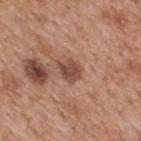<record>
  <biopsy_status>not biopsied; imaged during a skin examination</biopsy_status>
  <patient>
    <sex>male</sex>
    <age_approx>65</age_approx>
  </patient>
  <automated_metrics>
    <border_irregularity_0_10>2.5</border_irregularity_0_10>
    <peripheral_color_asymmetry>1.0</peripheral_color_asymmetry>
    <nevus_likeness_0_100>50</nevus_likeness_0_100>
  </automated_metrics>
  <lighting>white-light</lighting>
  <image>
    <source>total-body photography crop</source>
    <field_of_view_mm>15</field_of_view_mm>
  </image>
  <site>mid back</site>
</record>